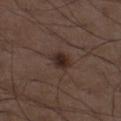A male patient about 50 years old. An algorithmic analysis of the crop reported a mean CIELAB color near L≈27 a*≈15 b*≈20, a lesion–skin lightness drop of about 9, and a normalized lesion–skin contrast near 10. It also reported an automated nevus-likeness rating near 95 out of 100. This is a white-light tile. About 3 mm across. This image is a 15 mm lesion crop taken from a total-body photograph. On the left thigh.